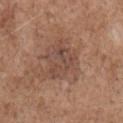The lesion was tiled from a total-body skin photograph and was not biopsied.
A roughly 15 mm field-of-view crop from a total-body skin photograph.
On the right upper arm.
An algorithmic analysis of the crop reported border irregularity of about 6.5 on a 0–10 scale, a within-lesion color-variation index near 4.5/10, and peripheral color asymmetry of about 1.5.
Longest diameter approximately 4 mm.
A male patient approximately 70 years of age.
Captured under white-light illumination.